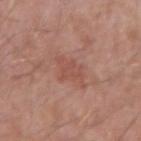Impression:
The lesion was tiled from a total-body skin photograph and was not biopsied.
Clinical summary:
The subject is a male aged 63–67. A lesion tile, about 15 mm wide, cut from a 3D total-body photograph. An algorithmic analysis of the crop reported a shape eccentricity near 0.7 and a shape-asymmetry score of about 0.3 (0 = symmetric). And it measured a mean CIELAB color near L≈51 a*≈24 b*≈27 and a lesion-to-skin contrast of about 5 (normalized; higher = more distinct). And it measured a border-irregularity index near 5/10 and a peripheral color-asymmetry measure near 0.5. Measured at roughly 3.5 mm in maximum diameter. Located on the right upper arm.